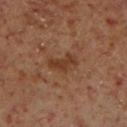Imaged during a routine full-body skin examination; the lesion was not biopsied and no histopathology is available.
This is a cross-polarized tile.
A male patient roughly 60 years of age.
The lesion is on the right lower leg.
The recorded lesion diameter is about 3.5 mm.
Cropped from a whole-body photographic skin survey; the tile spans about 15 mm.